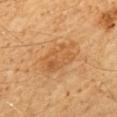  biopsy_status: not biopsied; imaged during a skin examination
  image:
    source: total-body photography crop
    field_of_view_mm: 15
  lesion_size:
    long_diameter_mm_approx: 5.0
  patient:
    sex: male
    age_approx: 60
  lighting: cross-polarized
  site: mid back
  automated_metrics:
    area_mm2_approx: 12.0
    eccentricity: 0.8
    shape_asymmetry: 0.2
    cielab_L: 47
    cielab_a: 19
    cielab_b: 36
    vs_skin_darker_L: 7.0